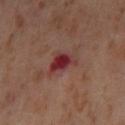Captured during whole-body skin photography for melanoma surveillance; the lesion was not biopsied. A region of skin cropped from a whole-body photographic capture, roughly 15 mm wide. The lesion is located on the left thigh. The lesion's longest dimension is about 3 mm. Captured under cross-polarized illumination. A female subject in their mid-50s.